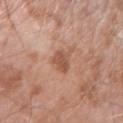Recorded during total-body skin imaging; not selected for excision or biopsy.
The total-body-photography lesion software estimated a symmetry-axis asymmetry near 0.25. The analysis additionally found a mean CIELAB color near L≈53 a*≈23 b*≈31. It also reported border irregularity of about 2.5 on a 0–10 scale and a within-lesion color-variation index near 2.5/10.
Longest diameter approximately 3 mm.
A male patient aged approximately 75.
On the right upper arm.
This image is a 15 mm lesion crop taken from a total-body photograph.